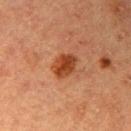diameter: ≈3.5 mm
lighting: cross-polarized illumination
patient: female, aged 68–72
image source: total-body-photography crop, ~15 mm field of view
body site: the arm
automated metrics: a footprint of about 7.5 mm², an outline eccentricity of about 0.65 (0 = round, 1 = elongated), and a shape-asymmetry score of about 0.15 (0 = symmetric); an automated nevus-likeness rating near 95 out of 100 and lesion-presence confidence of about 100/100